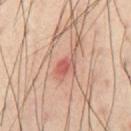{"biopsy_status": "not biopsied; imaged during a skin examination", "site": "right thigh", "image": {"source": "total-body photography crop", "field_of_view_mm": 15}, "lesion_size": {"long_diameter_mm_approx": 3.0}, "patient": {"sex": "male", "age_approx": 55}, "lighting": "cross-polarized"}